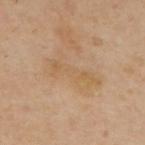notes: catalogued during a skin exam; not biopsied
size: ~6 mm (longest diameter)
image: ~15 mm tile from a whole-body skin photo
tile lighting: cross-polarized illumination
subject: male, aged 63 to 67
site: the upper back